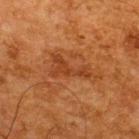Assessment: Imaged during a routine full-body skin examination; the lesion was not biopsied and no histopathology is available. Image and clinical context: Cropped from a total-body skin-imaging series; the visible field is about 15 mm. The tile uses cross-polarized illumination. Located on the upper back. A male patient, aged around 65.